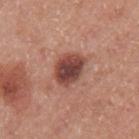follow-up — imaged on a skin check; not biopsied